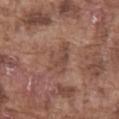<tbp_lesion>
  <biopsy_status>not biopsied; imaged during a skin examination</biopsy_status>
  <lighting>white-light</lighting>
  <patient>
    <sex>male</sex>
    <age_approx>75</age_approx>
  </patient>
  <lesion_size>
    <long_diameter_mm_approx>4.0</long_diameter_mm_approx>
  </lesion_size>
  <automated_metrics>
    <area_mm2_approx>7.5</area_mm2_approx>
    <eccentricity>0.8</eccentricity>
    <shape_asymmetry>0.35</shape_asymmetry>
    <cielab_L>46</cielab_L>
    <cielab_a>19</cielab_a>
    <cielab_b>26</cielab_b>
    <vs_skin_darker_L>7.0</vs_skin_darker_L>
    <vs_skin_contrast_norm>5.5</vs_skin_contrast_norm>
    <border_irregularity_0_10>4.5</border_irregularity_0_10>
    <peripheral_color_asymmetry>1.0</peripheral_color_asymmetry>
  </automated_metrics>
  <site>abdomen</site>
  <image>
    <source>total-body photography crop</source>
    <field_of_view_mm>15</field_of_view_mm>
  </image>
</tbp_lesion>